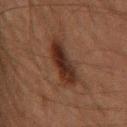notes: total-body-photography surveillance lesion; no biopsy | patient: male, aged approximately 60 | size: ~5 mm (longest diameter) | body site: the back | image: total-body-photography crop, ~15 mm field of view | TBP lesion metrics: a lesion–skin lightness drop of about 10 and a normalized border contrast of about 11.5; border irregularity of about 3 on a 0–10 scale and a within-lesion color-variation index near 4.5/10.